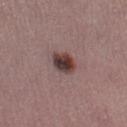{
  "biopsy_status": "not biopsied; imaged during a skin examination",
  "image": {
    "source": "total-body photography crop",
    "field_of_view_mm": 15
  },
  "site": "left thigh",
  "lighting": "white-light",
  "automated_metrics": {
    "cielab_L": 38,
    "cielab_a": 18,
    "cielab_b": 18,
    "vs_skin_darker_L": 15.0,
    "vs_skin_contrast_norm": 12.0,
    "color_variation_0_10": 6.5,
    "peripheral_color_asymmetry": 2.0,
    "nevus_likeness_0_100": 90,
    "lesion_detection_confidence_0_100": 100
  },
  "patient": {
    "sex": "female",
    "age_approx": 25
  },
  "lesion_size": {
    "long_diameter_mm_approx": 2.5
  }
}